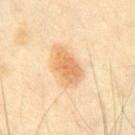notes = imaged on a skin check; not biopsied
patient = male, aged 63–67
location = the abdomen
imaging modality = ~15 mm tile from a whole-body skin photo
tile lighting = cross-polarized illumination
size = about 4.5 mm